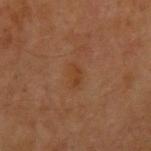<case>
<biopsy_status>not biopsied; imaged during a skin examination</biopsy_status>
<lesion_size>
  <long_diameter_mm_approx>2.5</long_diameter_mm_approx>
</lesion_size>
<image>
  <source>total-body photography crop</source>
  <field_of_view_mm>15</field_of_view_mm>
</image>
<lighting>cross-polarized</lighting>
<patient>
  <sex>male</sex>
  <age_approx>60</age_approx>
</patient>
<site>right upper arm</site>
</case>